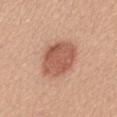Notes:
• follow-up — no biopsy performed (imaged during a skin exam)
• diameter — ~5.5 mm (longest diameter)
• illumination — white-light illumination
• anatomic site — the mid back
• subject — female, aged 38 to 42
• image source — ~15 mm tile from a whole-body skin photo